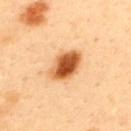The lesion was photographed on a routine skin check and not biopsied; there is no pathology result.
A male patient aged 38 to 42.
A 15 mm crop from a total-body photograph taken for skin-cancer surveillance.
From the upper back.